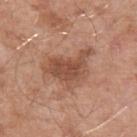Case summary:
* follow-up — imaged on a skin check; not biopsied
* imaging modality — 15 mm crop, total-body photography
* location — the left upper arm
* illumination — white-light illumination
* subject — male, aged 53–57